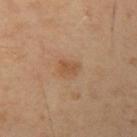Part of a total-body skin-imaging series; this lesion was reviewed on a skin check and was not flagged for biopsy. Cropped from a whole-body photographic skin survey; the tile spans about 15 mm. Captured under cross-polarized illumination. On the arm. The subject is a male roughly 40 years of age. Measured at roughly 2.5 mm in maximum diameter. Automated tile analysis of the lesion measured an area of roughly 4 mm², an eccentricity of roughly 0.7, and a symmetry-axis asymmetry near 0.25. The analysis additionally found a border-irregularity rating of about 2/10, a color-variation rating of about 2/10, and radial color variation of about 1.